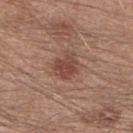Captured during whole-body skin photography for melanoma surveillance; the lesion was not biopsied. A male subject, aged approximately 40. The lesion is on the right forearm. A 15 mm close-up extracted from a 3D total-body photography capture.